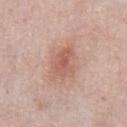Assessment:
This lesion was catalogued during total-body skin photography and was not selected for biopsy.
Background:
The total-body-photography lesion software estimated a lesion area of about 10 mm² and an outline eccentricity of about 0.7 (0 = round, 1 = elongated). The software also gave a normalized border contrast of about 6.5. The analysis additionally found a classifier nevus-likeness of about 60/100. Captured under white-light illumination. The subject is a male about 55 years old. Cropped from a total-body skin-imaging series; the visible field is about 15 mm. The lesion is on the abdomen.